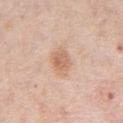| key | value |
|---|---|
| site | the chest |
| patient | male, approximately 75 years of age |
| image source | 15 mm crop, total-body photography |
| automated lesion analysis | a footprint of about 7 mm²; a mean CIELAB color near L≈65 a*≈20 b*≈32, a lesion–skin lightness drop of about 9, and a normalized border contrast of about 6.5; a nevus-likeness score of about 65/100 and lesion-presence confidence of about 100/100 |
| lighting | white-light |
| diameter | about 3.5 mm |